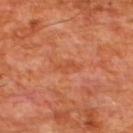patient: male, aged around 65
acquisition: 15 mm crop, total-body photography
illumination: cross-polarized
automated metrics: a lesion–skin lightness drop of about 6 and a normalized lesion–skin contrast near 5
size: ≈2.5 mm
anatomic site: the upper back